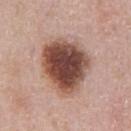Part of a total-body skin-imaging series; this lesion was reviewed on a skin check and was not flagged for biopsy.
A male patient in their 60s.
Automated image analysis of the tile measured an area of roughly 29 mm², an eccentricity of roughly 0.5, and two-axis asymmetry of about 0.2. And it measured a lesion color around L≈47 a*≈21 b*≈25 in CIELAB, roughly 20 lightness units darker than nearby skin, and a normalized lesion–skin contrast near 13.
Cropped from a total-body skin-imaging series; the visible field is about 15 mm.
Located on the abdomen.